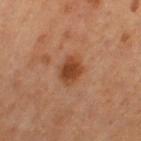No biopsy was performed on this lesion — it was imaged during a full skin examination and was not determined to be concerning. The recorded lesion diameter is about 3.5 mm. The subject is a female approximately 60 years of age. From the left thigh. A 15 mm close-up tile from a total-body photography series done for melanoma screening. An algorithmic analysis of the crop reported a footprint of about 7.5 mm² and an outline eccentricity of about 0.7 (0 = round, 1 = elongated). The software also gave a border-irregularity rating of about 2/10, a color-variation rating of about 3.5/10, and radial color variation of about 1. The analysis additionally found a nevus-likeness score of about 95/100 and a detector confidence of about 100 out of 100 that the crop contains a lesion. The tile uses cross-polarized illumination.